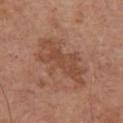notes = total-body-photography surveillance lesion; no biopsy | body site = the front of the torso | automated lesion analysis = a mean CIELAB color near L≈48 a*≈21 b*≈30, about 8 CIELAB-L* units darker than the surrounding skin, and a normalized lesion–skin contrast near 6 | illumination = white-light illumination | size = ~7.5 mm (longest diameter) | subject = male, in their mid- to late 70s | imaging modality = total-body-photography crop, ~15 mm field of view.